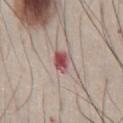• biopsy status — total-body-photography surveillance lesion; no biopsy
• illumination — white-light illumination
• automated metrics — an area of roughly 5 mm², an outline eccentricity of about 0.65 (0 = round, 1 = elongated), and a symmetry-axis asymmetry near 0.25; an average lesion color of about L≈52 a*≈26 b*≈20 (CIELAB), roughly 14 lightness units darker than nearby skin, and a normalized lesion–skin contrast near 9.5; a border-irregularity rating of about 2.5/10 and a color-variation rating of about 5/10; a classifier nevus-likeness of about 0/100 and lesion-presence confidence of about 100/100
• image — 15 mm crop, total-body photography
• subject — male, aged around 45
• body site — the front of the torso
• lesion diameter — about 3 mm